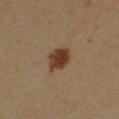Assessment:
This lesion was catalogued during total-body skin photography and was not selected for biopsy.
Clinical summary:
From the right upper arm. The total-body-photography lesion software estimated an eccentricity of roughly 0.55 and a symmetry-axis asymmetry near 0.15. It also reported a lesion color around L≈32 a*≈17 b*≈27 in CIELAB, a lesion–skin lightness drop of about 11, and a normalized lesion–skin contrast near 11. The analysis additionally found a border-irregularity rating of about 2/10 and internal color variation of about 4 on a 0–10 scale. And it measured a nevus-likeness score of about 100/100 and a detector confidence of about 100 out of 100 that the crop contains a lesion. Captured under cross-polarized illumination. Cropped from a whole-body photographic skin survey; the tile spans about 15 mm. A female subject, aged 18–22. Approximately 3 mm at its widest.